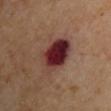Q: Was a biopsy performed?
A: no biopsy performed (imaged during a skin exam)
Q: What is the imaging modality?
A: ~15 mm crop, total-body skin-cancer survey
Q: Where on the body is the lesion?
A: the right upper arm
Q: How large is the lesion?
A: ~5.5 mm (longest diameter)
Q: What did automated image analysis measure?
A: a lesion color around L≈30 a*≈25 b*≈20 in CIELAB and a lesion-to-skin contrast of about 15.5 (normalized; higher = more distinct)
Q: How was the tile lit?
A: cross-polarized
Q: What are the patient's age and sex?
A: male, aged approximately 70